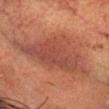Part of a total-body skin-imaging series; this lesion was reviewed on a skin check and was not flagged for biopsy. A male patient, in their 60s. Measured at roughly 10 mm in maximum diameter. A roughly 15 mm field-of-view crop from a total-body skin photograph. Imaged with cross-polarized lighting. On the head or neck.